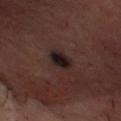subject = male, in their mid- to late 50s
size = ≈3.5 mm
anatomic site = the head or neck
illumination = cross-polarized
imaging modality = 15 mm crop, total-body photography
image-analysis metrics = a border-irregularity rating of about 2/10